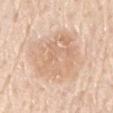{
  "biopsy_status": "not biopsied; imaged during a skin examination",
  "site": "right upper arm",
  "patient": {
    "sex": "male",
    "age_approx": 80
  },
  "image": {
    "source": "total-body photography crop",
    "field_of_view_mm": 15
  }
}